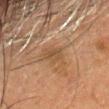notes = imaged on a skin check; not biopsied | automated lesion analysis = a lesion color around L≈41 a*≈15 b*≈30 in CIELAB and a normalized lesion–skin contrast near 5.5; a border-irregularity rating of about 3.5/10, a within-lesion color-variation index near 2/10, and radial color variation of about 1 | tile lighting = cross-polarized illumination | body site = the head or neck | lesion diameter = ~2.5 mm (longest diameter) | patient = female, aged 68–72 | imaging modality = ~15 mm crop, total-body skin-cancer survey.